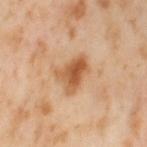This lesion was catalogued during total-body skin photography and was not selected for biopsy. Captured under cross-polarized illumination. The lesion is located on the left thigh. The subject is a female aged 53 to 57. About 4.5 mm across. A 15 mm close-up tile from a total-body photography series done for melanoma screening.